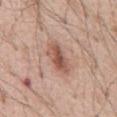notes: imaged on a skin check; not biopsied
illumination: white-light illumination
acquisition: ~15 mm tile from a whole-body skin photo
site: the abdomen
patient: male, in their mid- to late 50s
lesion size: ≈4.5 mm